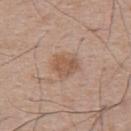The lesion was tiled from a total-body skin photograph and was not biopsied. The lesion's longest dimension is about 3.5 mm. Located on the upper back. The total-body-photography lesion software estimated a footprint of about 8.5 mm², an outline eccentricity of about 0.55 (0 = round, 1 = elongated), and a shape-asymmetry score of about 0.2 (0 = symmetric). It also reported roughly 8 lightness units darker than nearby skin. The software also gave a lesion-detection confidence of about 100/100. The subject is a male aged around 65. This is a white-light tile. A 15 mm close-up extracted from a 3D total-body photography capture.